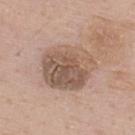The lesion was tiled from a total-body skin photograph and was not biopsied. The subject is a male in their mid- to late 50s. An algorithmic analysis of the crop reported a lesion area of about 25 mm², a shape eccentricity near 0.45, and a symmetry-axis asymmetry near 0.15. And it measured a lesion color around L≈55 a*≈16 b*≈27 in CIELAB, roughly 11 lightness units darker than nearby skin, and a normalized lesion–skin contrast near 7.5. A lesion tile, about 15 mm wide, cut from a 3D total-body photograph. From the upper back. About 6 mm across. The tile uses white-light illumination.